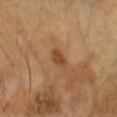<tbp_lesion>
  <biopsy_status>not biopsied; imaged during a skin examination</biopsy_status>
  <image>
    <source>total-body photography crop</source>
    <field_of_view_mm>15</field_of_view_mm>
  </image>
  <patient>
    <sex>male</sex>
    <age_approx>45</age_approx>
  </patient>
  <site>head or neck</site>
</tbp_lesion>